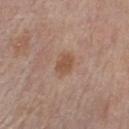image:
  source: total-body photography crop
  field_of_view_mm: 15
lesion_size:
  long_diameter_mm_approx: 3.0
site: front of the torso
patient:
  sex: male
  age_approx: 80
automated_metrics:
  border_irregularity_0_10: 2.0
  color_variation_0_10: 1.5
  peripheral_color_asymmetry: 0.5